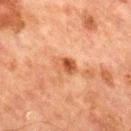Q: How was this image acquired?
A: 15 mm crop, total-body photography
Q: Lesion location?
A: the front of the torso
Q: Who is the patient?
A: male, approximately 65 years of age